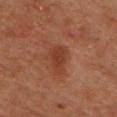Findings:
• workup — catalogued during a skin exam; not biopsied
• illumination — cross-polarized illumination
• location — the back
• patient — male, aged approximately 60
• lesion diameter — about 4 mm
• image source — total-body-photography crop, ~15 mm field of view